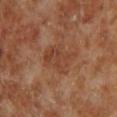Impression: The lesion was tiled from a total-body skin photograph and was not biopsied. Acquisition and patient details: A lesion tile, about 15 mm wide, cut from a 3D total-body photograph. On the left lower leg. The patient is a male approximately 70 years of age. This is a cross-polarized tile.